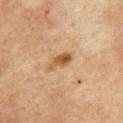This is a cross-polarized tile.
Located on the chest.
A 15 mm close-up tile from a total-body photography series done for melanoma screening.
A male patient, roughly 65 years of age.